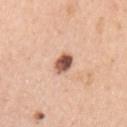Assessment:
Part of a total-body skin-imaging series; this lesion was reviewed on a skin check and was not flagged for biopsy.
Context:
A female patient about 60 years old. Approximately 2.5 mm at its widest. The lesion-visualizer software estimated a mean CIELAB color near L≈55 a*≈23 b*≈29 and about 20 CIELAB-L* units darker than the surrounding skin. And it measured a classifier nevus-likeness of about 95/100. A 15 mm close-up tile from a total-body photography series done for melanoma screening. Captured under white-light illumination. The lesion is on the left upper arm.